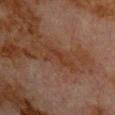Case summary:
• notes: catalogued during a skin exam; not biopsied
• automated metrics: a footprint of about 5 mm², an outline eccentricity of about 0.85 (0 = round, 1 = elongated), and a symmetry-axis asymmetry near 0.55; a normalized lesion–skin contrast near 5.5; a border-irregularity index near 7.5/10, a color-variation rating of about 1/10, and a peripheral color-asymmetry measure near 0.5; a classifier nevus-likeness of about 0/100
• patient: male, aged 78–82
• anatomic site: the chest
• illumination: cross-polarized
• image source: ~15 mm crop, total-body skin-cancer survey